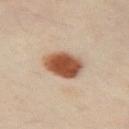The lesion was photographed on a routine skin check and not biopsied; there is no pathology result.